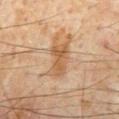notes = no biopsy performed (imaged during a skin exam)